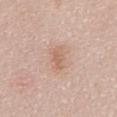follow-up=no biopsy performed (imaged during a skin exam)
size=about 3 mm
patient=male, aged 63–67
anatomic site=the chest
acquisition=total-body-photography crop, ~15 mm field of view